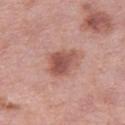follow-up: no biopsy performed (imaged during a skin exam) | image-analysis metrics: a lesion area of about 9.5 mm²; a classifier nevus-likeness of about 75/100 | image: total-body-photography crop, ~15 mm field of view | size: ≈3.5 mm | patient: female, approximately 40 years of age | anatomic site: the left thigh.